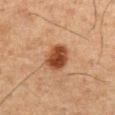{"biopsy_status": "not biopsied; imaged during a skin examination", "lighting": "cross-polarized", "patient": {"sex": "male", "age_approx": 75}, "site": "abdomen", "lesion_size": {"long_diameter_mm_approx": 3.5}, "image": {"source": "total-body photography crop", "field_of_view_mm": 15}}